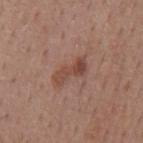A lesion tile, about 15 mm wide, cut from a 3D total-body photograph.
Captured under white-light illumination.
On the mid back.
The subject is a male aged 58 to 62.
Approximately 4 mm at its widest.